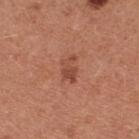Findings:
– subject: female, in their mid- to late 30s
– automated metrics: an area of roughly 4.5 mm², an outline eccentricity of about 0.8 (0 = round, 1 = elongated), and two-axis asymmetry of about 0.35; a lesion–skin lightness drop of about 9 and a lesion-to-skin contrast of about 6.5 (normalized; higher = more distinct); a within-lesion color-variation index near 1/10 and radial color variation of about 0.5; a classifier nevus-likeness of about 20/100 and a detector confidence of about 100 out of 100 that the crop contains a lesion
– diameter: ≈3 mm
– imaging modality: ~15 mm tile from a whole-body skin photo
– illumination: white-light illumination
– location: the front of the torso